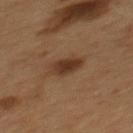Assessment: The lesion was tiled from a total-body skin photograph and was not biopsied. Context: A male subject approximately 55 years of age. A 15 mm close-up tile from a total-body photography series done for melanoma screening. The lesion's longest dimension is about 3 mm. The tile uses cross-polarized illumination. From the mid back.